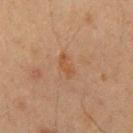{
  "biopsy_status": "not biopsied; imaged during a skin examination",
  "automated_metrics": {
    "cielab_L": 38,
    "cielab_a": 17,
    "cielab_b": 28,
    "vs_skin_contrast_norm": 6.0,
    "border_irregularity_0_10": 3.0,
    "color_variation_0_10": 1.0,
    "peripheral_color_asymmetry": 0.5
  },
  "patient": {
    "sex": "male",
    "age_approx": 50
  },
  "lesion_size": {
    "long_diameter_mm_approx": 3.0
  },
  "site": "chest",
  "image": {
    "source": "total-body photography crop",
    "field_of_view_mm": 15
  },
  "lighting": "cross-polarized"
}